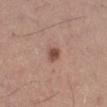Imaged during a routine full-body skin examination; the lesion was not biopsied and no histopathology is available. Captured under white-light illumination. Approximately 2 mm at its widest. Automated image analysis of the tile measured a footprint of about 3 mm², a shape eccentricity near 0.5, and two-axis asymmetry of about 0.15. And it measured a detector confidence of about 100 out of 100 that the crop contains a lesion. A 15 mm close-up extracted from a 3D total-body photography capture. From the leg. A male patient, approximately 60 years of age.